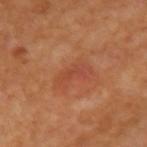– patient — female, in their mid-50s
– image source — 15 mm crop, total-body photography
– tile lighting — cross-polarized illumination
– lesion diameter — about 3 mm
– body site — the arm